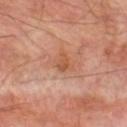<tbp_lesion>
  <biopsy_status>not biopsied; imaged during a skin examination</biopsy_status>
  <image>
    <source>total-body photography crop</source>
    <field_of_view_mm>15</field_of_view_mm>
  </image>
  <lighting>cross-polarized</lighting>
  <patient>
    <sex>male</sex>
    <age_approx>70</age_approx>
  </patient>
  <lesion_size>
    <long_diameter_mm_approx>2.5</long_diameter_mm_approx>
  </lesion_size>
  <site>left thigh</site>
</tbp_lesion>